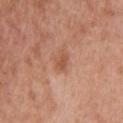Acquisition and patient details: On the back. The patient is a male approximately 60 years of age. The recorded lesion diameter is about 2.5 mm. Imaged with white-light lighting. A roughly 15 mm field-of-view crop from a total-body skin photograph.